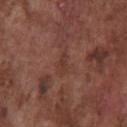biopsy_status: not biopsied; imaged during a skin examination
site: chest
lighting: white-light
lesion_size:
  long_diameter_mm_approx: 2.5
image:
  source: total-body photography crop
  field_of_view_mm: 15
patient:
  sex: male
  age_approx: 75